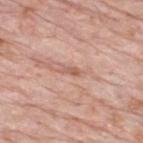The lesion was tiled from a total-body skin photograph and was not biopsied.